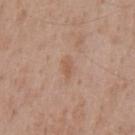The lesion was tiled from a total-body skin photograph and was not biopsied. A close-up tile cropped from a whole-body skin photograph, about 15 mm across. Located on the chest. About 3 mm across. Automated tile analysis of the lesion measured a symmetry-axis asymmetry near 0.25. It also reported a lesion color around L≈56 a*≈19 b*≈30 in CIELAB, about 7 CIELAB-L* units darker than the surrounding skin, and a normalized lesion–skin contrast near 5.5. The software also gave internal color variation of about 1 on a 0–10 scale. It also reported lesion-presence confidence of about 100/100. Imaged with white-light lighting. A male patient, aged approximately 45.